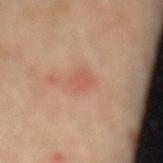{"biopsy_status": "not biopsied; imaged during a skin examination", "automated_metrics": {"nevus_likeness_0_100": 0, "lesion_detection_confidence_0_100": 100}, "site": "lower back", "lesion_size": {"long_diameter_mm_approx": 2.5}, "image": {"source": "total-body photography crop", "field_of_view_mm": 15}, "patient": {"sex": "male", "age_approx": 65}}